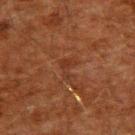biopsy_status: not biopsied; imaged during a skin examination
site: upper back
automated_metrics:
  color_variation_0_10: 0.0
  peripheral_color_asymmetry: 0.0
lighting: cross-polarized
image:
  source: total-body photography crop
  field_of_view_mm: 15
patient:
  sex: male
  age_approx: 60
lesion_size:
  long_diameter_mm_approx: 2.5